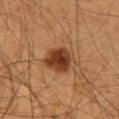Case summary:
- notes: no biopsy performed (imaged during a skin exam)
- subject: male, about 65 years old
- anatomic site: the lower back
- size: about 3.5 mm
- acquisition: ~15 mm tile from a whole-body skin photo